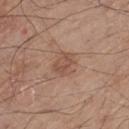No biopsy was performed on this lesion — it was imaged during a full skin examination and was not determined to be concerning. From the left thigh. The patient is a male aged 78–82. This image is a 15 mm lesion crop taken from a total-body photograph. The lesion's longest dimension is about 3 mm. The lesion-visualizer software estimated a lesion color around L≈51 a*≈20 b*≈28 in CIELAB and roughly 8 lightness units darker than nearby skin. And it measured a border-irregularity index near 2/10, a within-lesion color-variation index near 3/10, and peripheral color asymmetry of about 1.